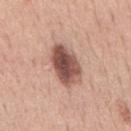Recorded during total-body skin imaging; not selected for excision or biopsy. A male subject roughly 50 years of age. Automated tile analysis of the lesion measured a lesion area of about 13 mm², a shape eccentricity near 0.85, and two-axis asymmetry of about 0.15. And it measured a detector confidence of about 100 out of 100 that the crop contains a lesion. The lesion is on the mid back. A roughly 15 mm field-of-view crop from a total-body skin photograph. About 5.5 mm across.